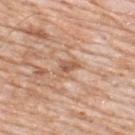Part of a total-body skin-imaging series; this lesion was reviewed on a skin check and was not flagged for biopsy. A roughly 15 mm field-of-view crop from a total-body skin photograph. A male subject, roughly 80 years of age. Approximately 2.5 mm at its widest. The lesion is on the upper back. Imaged with white-light lighting.